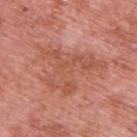Assessment: Part of a total-body skin-imaging series; this lesion was reviewed on a skin check and was not flagged for biopsy. Acquisition and patient details: This image is a 15 mm lesion crop taken from a total-body photograph. From the upper back. A male patient roughly 70 years of age.